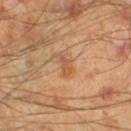The lesion was photographed on a routine skin check and not biopsied; there is no pathology result. The patient is a male aged 43–47. About 3 mm across. This image is a 15 mm lesion crop taken from a total-body photograph. Automated image analysis of the tile measured an area of roughly 3 mm², an eccentricity of roughly 0.9, and a symmetry-axis asymmetry near 0.45. The analysis additionally found a mean CIELAB color near L≈54 a*≈22 b*≈37, roughly 8 lightness units darker than nearby skin, and a normalized lesion–skin contrast near 6. The software also gave internal color variation of about 0.5 on a 0–10 scale and peripheral color asymmetry of about 0. It also reported an automated nevus-likeness rating near 0 out of 100. The lesion is on the left lower leg.